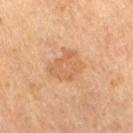The lesion was photographed on a routine skin check and not biopsied; there is no pathology result. On the right thigh. The lesion's longest dimension is about 4.5 mm. Cropped from a total-body skin-imaging series; the visible field is about 15 mm. Imaged with cross-polarized lighting. The patient is a female aged approximately 70.